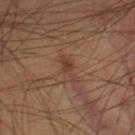{
  "biopsy_status": "not biopsied; imaged during a skin examination",
  "lesion_size": {
    "long_diameter_mm_approx": 3.5
  },
  "site": "right lower leg",
  "image": {
    "source": "total-body photography crop",
    "field_of_view_mm": 15
  },
  "patient": {
    "sex": "male",
    "age_approx": 40
  }
}